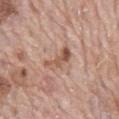Q: Is there a histopathology result?
A: catalogued during a skin exam; not biopsied
Q: How large is the lesion?
A: about 4 mm
Q: What are the patient's age and sex?
A: male, aged around 70
Q: What is the anatomic site?
A: the mid back
Q: What kind of image is this?
A: ~15 mm crop, total-body skin-cancer survey